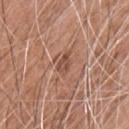<lesion>
  <biopsy_status>not biopsied; imaged during a skin examination</biopsy_status>
  <automated_metrics>
    <vs_skin_darker_L>9.0</vs_skin_darker_L>
    <vs_skin_contrast_norm>6.5</vs_skin_contrast_norm>
    <nevus_likeness_0_100>0</nevus_likeness_0_100>
    <lesion_detection_confidence_0_100>80</lesion_detection_confidence_0_100>
  </automated_metrics>
  <site>chest</site>
  <lesion_size>
    <long_diameter_mm_approx>2.5</long_diameter_mm_approx>
  </lesion_size>
  <image>
    <source>total-body photography crop</source>
    <field_of_view_mm>15</field_of_view_mm>
  </image>
  <lighting>white-light</lighting>
  <patient>
    <sex>male</sex>
    <age_approx>60</age_approx>
  </patient>
</lesion>